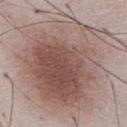| field | value |
|---|---|
| follow-up | catalogued during a skin exam; not biopsied |
| illumination | white-light illumination |
| size | ~15 mm (longest diameter) |
| automated lesion analysis | a footprint of about 125 mm² and an eccentricity of roughly 0.5; a lesion color around L≈56 a*≈16 b*≈22 in CIELAB and roughly 11 lightness units darker than nearby skin; lesion-presence confidence of about 100/100 |
| image | ~15 mm crop, total-body skin-cancer survey |
| subject | male, aged around 50 |
| site | the abdomen |